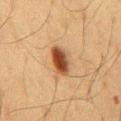{
  "biopsy_status": "not biopsied; imaged during a skin examination",
  "image": {
    "source": "total-body photography crop",
    "field_of_view_mm": 15
  },
  "patient": {
    "sex": "male",
    "age_approx": 60
  },
  "lesion_size": {
    "long_diameter_mm_approx": 3.5
  },
  "site": "chest"
}